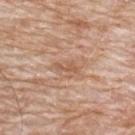The lesion was photographed on a routine skin check and not biopsied; there is no pathology result.
Approximately 3 mm at its widest.
An algorithmic analysis of the crop reported a border-irregularity rating of about 5.5/10, a color-variation rating of about 0/10, and radial color variation of about 0. The analysis additionally found a nevus-likeness score of about 0/100 and a lesion-detection confidence of about 90/100.
A 15 mm crop from a total-body photograph taken for skin-cancer surveillance.
On the upper back.
The subject is a male aged 78 to 82.
Imaged with white-light lighting.